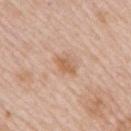This lesion was catalogued during total-body skin photography and was not selected for biopsy.
A female subject aged 43 to 47.
Longest diameter approximately 3 mm.
A 15 mm crop from a total-body photograph taken for skin-cancer surveillance.
The lesion-visualizer software estimated an average lesion color of about L≈61 a*≈20 b*≈34 (CIELAB), about 9 CIELAB-L* units darker than the surrounding skin, and a normalized lesion–skin contrast near 7. The software also gave a classifier nevus-likeness of about 5/100 and a lesion-detection confidence of about 100/100.
Located on the right upper arm.